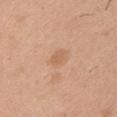| feature | finding |
|---|---|
| follow-up | imaged on a skin check; not biopsied |
| body site | the left upper arm |
| automated lesion analysis | border irregularity of about 2 on a 0–10 scale, a within-lesion color-variation index near 1.5/10, and peripheral color asymmetry of about 0.5; an automated nevus-likeness rating near 5 out of 100 |
| tile lighting | white-light |
| patient | male, roughly 40 years of age |
| image | ~15 mm tile from a whole-body skin photo |